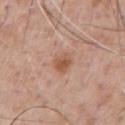notes: imaged on a skin check; not biopsied | image-analysis metrics: an area of roughly 4.5 mm², an eccentricity of roughly 0.55, and a shape-asymmetry score of about 0.25 (0 = symmetric); a lesion color around L≈55 a*≈22 b*≈31 in CIELAB; an automated nevus-likeness rating near 70 out of 100 | site: the chest | lighting: white-light | image source: ~15 mm tile from a whole-body skin photo | lesion diameter: ≈2.5 mm | patient: male, aged 58 to 62.